From the chest. The lesion-visualizer software estimated a lesion color around L≈51 a*≈23 b*≈30 in CIELAB, about 10 CIELAB-L* units darker than the surrounding skin, and a lesion-to-skin contrast of about 7 (normalized; higher = more distinct). The analysis additionally found border irregularity of about 1.5 on a 0–10 scale, a color-variation rating of about 2.5/10, and peripheral color asymmetry of about 1. The lesion's longest dimension is about 4 mm. A male subject about 65 years old. Cropped from a total-body skin-imaging series; the visible field is about 15 mm. This is a cross-polarized tile — 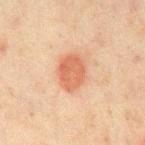Case summary:
* histopathologic diagnosis: a nodular basal cell carcinoma (malignant)